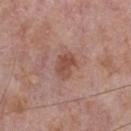No biopsy was performed on this lesion — it was imaged during a full skin examination and was not determined to be concerning. A male subject aged approximately 75. The tile uses white-light illumination. An algorithmic analysis of the crop reported an outline eccentricity of about 0.7 (0 = round, 1 = elongated). It also reported an average lesion color of about L≈49 a*≈23 b*≈27 (CIELAB), about 9 CIELAB-L* units darker than the surrounding skin, and a normalized border contrast of about 7. It also reported a border-irregularity index near 2.5/10 and a peripheral color-asymmetry measure near 1. And it measured an automated nevus-likeness rating near 35 out of 100 and lesion-presence confidence of about 100/100. Located on the chest. The lesion's longest dimension is about 3.5 mm. Cropped from a total-body skin-imaging series; the visible field is about 15 mm.